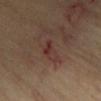The lesion was photographed on a routine skin check and not biopsied; there is no pathology result. The lesion is located on the chest. A male patient about 70 years old. The recorded lesion diameter is about 3 mm. The lesion-visualizer software estimated a mean CIELAB color near L≈32 a*≈19 b*≈21. Captured under cross-polarized illumination. Cropped from a total-body skin-imaging series; the visible field is about 15 mm.